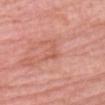Recorded during total-body skin imaging; not selected for excision or biopsy.
A 15 mm crop from a total-body photograph taken for skin-cancer surveillance.
The lesion-visualizer software estimated an area of roughly 3 mm² and a shape eccentricity near 0.7.
A female patient, roughly 60 years of age.
Captured under white-light illumination.
The lesion's longest dimension is about 2.5 mm.
From the head or neck.